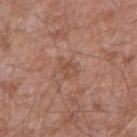Background:
A 15 mm close-up extracted from a 3D total-body photography capture. The lesion is on the arm. A male patient, in their mid-70s. An algorithmic analysis of the crop reported a border-irregularity index near 3.5/10, internal color variation of about 2 on a 0–10 scale, and peripheral color asymmetry of about 0.5. The recorded lesion diameter is about 3 mm. This is a white-light tile.